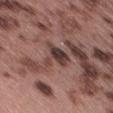Part of a total-body skin-imaging series; this lesion was reviewed on a skin check and was not flagged for biopsy.